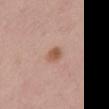Clinical summary: The recorded lesion diameter is about 2.5 mm. A 15 mm close-up extracted from a 3D total-body photography capture. The lesion is located on the mid back. The tile uses white-light illumination. A female patient, in their 40s.